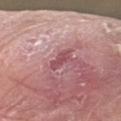The lesion was tiled from a total-body skin photograph and was not biopsied. Automated tile analysis of the lesion measured a lesion color around L≈50 a*≈28 b*≈18 in CIELAB, roughly 10 lightness units darker than nearby skin, and a normalized border contrast of about 7. And it measured border irregularity of about 3.5 on a 0–10 scale, internal color variation of about 1 on a 0–10 scale, and a peripheral color-asymmetry measure near 0. From the left forearm. The patient is a male aged 63 to 67. The recorded lesion diameter is about 3.5 mm. Cropped from a total-body skin-imaging series; the visible field is about 15 mm. The tile uses white-light illumination.